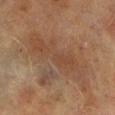Impression:
The lesion was tiled from a total-body skin photograph and was not biopsied.
Image and clinical context:
A male subject, aged 68–72. The lesion is on the right lower leg. Imaged with cross-polarized lighting. The lesion-visualizer software estimated a nevus-likeness score of about 0/100 and lesion-presence confidence of about 85/100. Cropped from a total-body skin-imaging series; the visible field is about 15 mm.